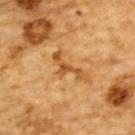Imaged during a routine full-body skin examination; the lesion was not biopsied and no histopathology is available.
The patient is a male aged around 85 or older.
Approximately 4.5 mm at its widest.
This image is a 15 mm lesion crop taken from a total-body photograph.
On the upper back.
The tile uses cross-polarized illumination.